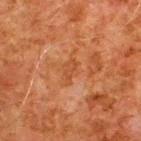Recorded during total-body skin imaging; not selected for excision or biopsy.